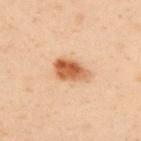| field | value |
|---|---|
| imaging modality | total-body-photography crop, ~15 mm field of view |
| lesion size | ~4 mm (longest diameter) |
| automated lesion analysis | a border-irregularity index near 2.5/10 and a peripheral color-asymmetry measure near 2 |
| subject | male, aged 48 to 52 |
| tile lighting | cross-polarized illumination |
| body site | the upper back |